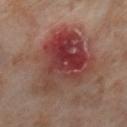A 15 mm crop from a total-body photograph taken for skin-cancer surveillance. The patient is a female roughly 55 years of age. On the right lower leg. The total-body-photography lesion software estimated an average lesion color of about L≈41 a*≈26 b*≈24 (CIELAB), a lesion–skin lightness drop of about 11, and a lesion-to-skin contrast of about 9 (normalized; higher = more distinct). And it measured an automated nevus-likeness rating near 0 out of 100 and lesion-presence confidence of about 100/100. This is a cross-polarized tile.